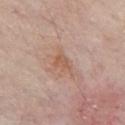This lesion was catalogued during total-body skin photography and was not selected for biopsy. This is a white-light tile. This image is a 15 mm lesion crop taken from a total-body photograph. An algorithmic analysis of the crop reported a shape eccentricity near 0.7 and a symmetry-axis asymmetry near 0.45. The software also gave a border-irregularity index near 4/10, a color-variation rating of about 1.5/10, and a peripheral color-asymmetry measure near 0.5. It also reported a classifier nevus-likeness of about 0/100 and a lesion-detection confidence of about 100/100. The patient is a male aged 83 or over. On the chest. Measured at roughly 2.5 mm in maximum diameter.